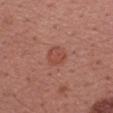Clinical impression:
Imaged during a routine full-body skin examination; the lesion was not biopsied and no histopathology is available.
Acquisition and patient details:
From the right forearm. Automated image analysis of the tile measured an average lesion color of about L≈48 a*≈27 b*≈29 (CIELAB) and a lesion–skin lightness drop of about 7. It also reported a border-irregularity index near 2/10, a within-lesion color-variation index near 2.5/10, and a peripheral color-asymmetry measure near 1. The analysis additionally found a classifier nevus-likeness of about 40/100 and a detector confidence of about 100 out of 100 that the crop contains a lesion. A female subject in their mid-50s. Cropped from a whole-body photographic skin survey; the tile spans about 15 mm. Longest diameter approximately 2.5 mm.